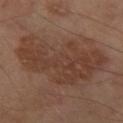biopsy_status: not biopsied; imaged during a skin examination
lighting: cross-polarized
patient:
  sex: male
  age_approx: 70
image:
  source: total-body photography crop
  field_of_view_mm: 15
lesion_size:
  long_diameter_mm_approx: 10.0
site: right thigh